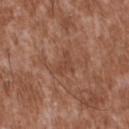Assessment:
Imaged during a routine full-body skin examination; the lesion was not biopsied and no histopathology is available.
Clinical summary:
Longest diameter approximately 3 mm. The lesion is located on the upper back. A male patient aged approximately 45. The tile uses white-light illumination. A region of skin cropped from a whole-body photographic capture, roughly 15 mm wide. An algorithmic analysis of the crop reported a lesion area of about 3 mm². The software also gave border irregularity of about 5.5 on a 0–10 scale, a within-lesion color-variation index near 0.5/10, and a peripheral color-asymmetry measure near 0.5.